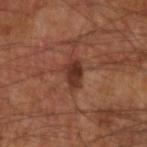Recorded during total-body skin imaging; not selected for excision or biopsy.